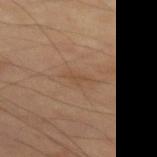workup = imaged on a skin check; not biopsied | site = the left thigh | lesion diameter = ≈2.5 mm | lighting = cross-polarized illumination | image = 15 mm crop, total-body photography | patient = male, roughly 70 years of age.